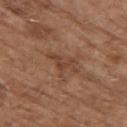Q: Was this lesion biopsied?
A: no biopsy performed (imaged during a skin exam)
Q: Lesion location?
A: the upper back
Q: Illumination type?
A: white-light illumination
Q: Patient demographics?
A: male, aged around 75
Q: Lesion size?
A: about 4 mm
Q: How was this image acquired?
A: 15 mm crop, total-body photography